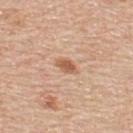Captured during whole-body skin photography for melanoma surveillance; the lesion was not biopsied. This is a white-light tile. Approximately 2.5 mm at its widest. Located on the upper back. The patient is a male aged 58–62. A 15 mm crop from a total-body photograph taken for skin-cancer surveillance.